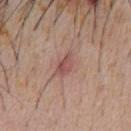Clinical impression: Recorded during total-body skin imaging; not selected for excision or biopsy. Background: Cropped from a total-body skin-imaging series; the visible field is about 15 mm. An algorithmic analysis of the crop reported a classifier nevus-likeness of about 10/100 and a lesion-detection confidence of about 100/100. A male patient, aged around 55. Longest diameter approximately 3 mm. Located on the front of the torso. Captured under white-light illumination.